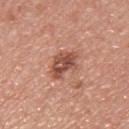Part of a total-body skin-imaging series; this lesion was reviewed on a skin check and was not flagged for biopsy. A male patient, aged 38–42. Automated tile analysis of the lesion measured an eccentricity of roughly 0.85 and two-axis asymmetry of about 0.25. The analysis additionally found a lesion color around L≈51 a*≈26 b*≈29 in CIELAB, about 13 CIELAB-L* units darker than the surrounding skin, and a normalized border contrast of about 9. The analysis additionally found border irregularity of about 3 on a 0–10 scale and a within-lesion color-variation index near 5.5/10. The software also gave an automated nevus-likeness rating near 30 out of 100. About 4.5 mm across. From the back. Captured under white-light illumination. A roughly 15 mm field-of-view crop from a total-body skin photograph.